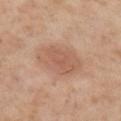anatomic site: the right upper arm
lesion diameter: about 5.5 mm
patient: male, approximately 55 years of age
tile lighting: cross-polarized
image source: ~15 mm tile from a whole-body skin photo